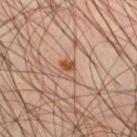{"lesion_size": {"long_diameter_mm_approx": 2.5}, "patient": {"sex": "male", "age_approx": 45}, "site": "right thigh", "lighting": "cross-polarized", "image": {"source": "total-body photography crop", "field_of_view_mm": 15}}